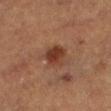Part of a total-body skin-imaging series; this lesion was reviewed on a skin check and was not flagged for biopsy.
An algorithmic analysis of the crop reported a symmetry-axis asymmetry near 0.2. It also reported a color-variation rating of about 3/10 and radial color variation of about 1. The analysis additionally found an automated nevus-likeness rating near 95 out of 100 and a detector confidence of about 100 out of 100 that the crop contains a lesion.
A male subject, in their mid-70s.
The lesion's longest dimension is about 3 mm.
From the leg.
This is a cross-polarized tile.
A 15 mm close-up extracted from a 3D total-body photography capture.